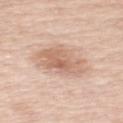Recorded during total-body skin imaging; not selected for excision or biopsy.
Cropped from a total-body skin-imaging series; the visible field is about 15 mm.
The recorded lesion diameter is about 5.5 mm.
Located on the left upper arm.
The patient is a female aged around 50.
The total-body-photography lesion software estimated a peripheral color-asymmetry measure near 1.5.